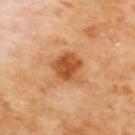Q: Was this lesion biopsied?
A: no biopsy performed (imaged during a skin exam)
Q: How was the tile lit?
A: cross-polarized
Q: Automated lesion metrics?
A: a footprint of about 9.5 mm², an eccentricity of roughly 0.45, and a shape-asymmetry score of about 0.3 (0 = symmetric); a mean CIELAB color near L≈55 a*≈28 b*≈43, roughly 13 lightness units darker than nearby skin, and a normalized lesion–skin contrast near 9; an automated nevus-likeness rating near 85 out of 100 and lesion-presence confidence of about 100/100
Q: What is the anatomic site?
A: the upper back
Q: How was this image acquired?
A: 15 mm crop, total-body photography
Q: How large is the lesion?
A: about 4 mm